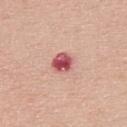The patient is a male aged approximately 45. Automated image analysis of the tile measured a mean CIELAB color near L≈58 a*≈30 b*≈23 and about 14 CIELAB-L* units darker than the surrounding skin. And it measured internal color variation of about 8.5 on a 0–10 scale and a peripheral color-asymmetry measure near 2.5. A 15 mm crop from a total-body photograph taken for skin-cancer surveillance. Imaged with white-light lighting. On the upper back. About 3.5 mm across.